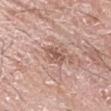biopsy status = imaged on a skin check; not biopsied